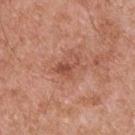Q: Was this lesion biopsied?
A: total-body-photography surveillance lesion; no biopsy
Q: Patient demographics?
A: male, aged 53–57
Q: What kind of image is this?
A: ~15 mm tile from a whole-body skin photo
Q: Lesion location?
A: the upper back
Q: What did automated image analysis measure?
A: border irregularity of about 6.5 on a 0–10 scale, a color-variation rating of about 2.5/10, and a peripheral color-asymmetry measure near 0.5; an automated nevus-likeness rating near 0 out of 100 and lesion-presence confidence of about 100/100
Q: What lighting was used for the tile?
A: white-light illumination